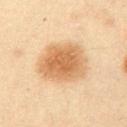<case>
<biopsy_status>not biopsied; imaged during a skin examination</biopsy_status>
<patient>
  <sex>male</sex>
  <age_approx>35</age_approx>
</patient>
<lighting>cross-polarized</lighting>
<automated_metrics>
  <area_mm2_approx>21.0</area_mm2_approx>
  <eccentricity>0.35</eccentricity>
  <shape_asymmetry>0.15</shape_asymmetry>
</automated_metrics>
<lesion_size>
  <long_diameter_mm_approx>5.5</long_diameter_mm_approx>
</lesion_size>
<site>left upper arm</site>
<image>
  <source>total-body photography crop</source>
  <field_of_view_mm>15</field_of_view_mm>
</image>
</case>